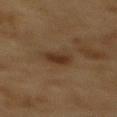| key | value |
|---|---|
| workup | imaged on a skin check; not biopsied |
| patient | male, in their mid-80s or older |
| body site | the mid back |
| automated metrics | a lesion-detection confidence of about 100/100 |
| imaging modality | ~15 mm crop, total-body skin-cancer survey |